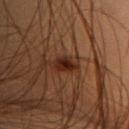The lesion was tiled from a total-body skin photograph and was not biopsied.
Imaged with cross-polarized lighting.
A female patient, about 40 years old.
Automated tile analysis of the lesion measured a footprint of about 4 mm², an outline eccentricity of about 0.8 (0 = round, 1 = elongated), and a symmetry-axis asymmetry near 0.35. The software also gave a mean CIELAB color near L≈20 a*≈18 b*≈23, about 9 CIELAB-L* units darker than the surrounding skin, and a normalized border contrast of about 11.
From the front of the torso.
A 15 mm close-up tile from a total-body photography series done for melanoma screening.
Measured at roughly 3 mm in maximum diameter.